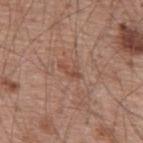follow-up = total-body-photography surveillance lesion; no biopsy
diameter = ~2.5 mm (longest diameter)
acquisition = 15 mm crop, total-body photography
body site = the upper back
TBP lesion metrics = an area of roughly 2.5 mm² and two-axis asymmetry of about 0.4; a nevus-likeness score of about 0/100 and a lesion-detection confidence of about 100/100
subject = male, aged approximately 55
lighting = white-light illumination